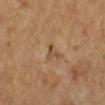Imaged during a routine full-body skin examination; the lesion was not biopsied and no histopathology is available.
The lesion's longest dimension is about 2.5 mm.
A patient about 60 years old.
Captured under cross-polarized illumination.
Cropped from a total-body skin-imaging series; the visible field is about 15 mm.
The lesion is on the arm.
An algorithmic analysis of the crop reported a lesion area of about 3 mm², a shape eccentricity near 0.75, and two-axis asymmetry of about 0.65. It also reported a mean CIELAB color near L≈49 a*≈18 b*≈33, roughly 8 lightness units darker than nearby skin, and a normalized border contrast of about 6. The analysis additionally found a border-irregularity rating of about 6.5/10, a within-lesion color-variation index near 0/10, and peripheral color asymmetry of about 0.